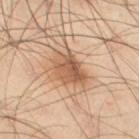Q: Was this lesion biopsied?
A: catalogued during a skin exam; not biopsied
Q: Illumination type?
A: cross-polarized illumination
Q: Lesion size?
A: ~4.5 mm (longest diameter)
Q: Where on the body is the lesion?
A: the right thigh
Q: Who is the patient?
A: male, aged 38 to 42
Q: How was this image acquired?
A: 15 mm crop, total-body photography
Q: Automated lesion metrics?
A: an area of roughly 11 mm², an eccentricity of roughly 0.6, and a symmetry-axis asymmetry near 0.25; border irregularity of about 3 on a 0–10 scale; a nevus-likeness score of about 65/100 and a detector confidence of about 100 out of 100 that the crop contains a lesion